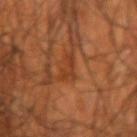Notes:
– notes — catalogued during a skin exam; not biopsied
– anatomic site — the left forearm
– lighting — cross-polarized illumination
– image source — ~15 mm crop, total-body skin-cancer survey
– size — ≈3 mm
– subject — male, aged 53–57